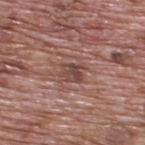Notes:
• workup · total-body-photography surveillance lesion; no biopsy
• site · the upper back
• acquisition · 15 mm crop, total-body photography
• subject · male, aged 68–72